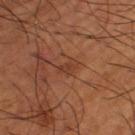notes = catalogued during a skin exam; not biopsied
image-analysis metrics = a border-irregularity index near 3/10 and a color-variation rating of about 1.5/10; a classifier nevus-likeness of about 0/100 and a lesion-detection confidence of about 100/100
lighting = cross-polarized illumination
image source = 15 mm crop, total-body photography
size = ≈3 mm
patient = male, approximately 65 years of age
location = the right lower leg